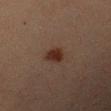notes — no biopsy performed (imaged during a skin exam) | imaging modality — 15 mm crop, total-body photography | location — the left upper arm | tile lighting — cross-polarized | lesion size — about 3 mm | patient — male, approximately 40 years of age.